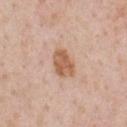Findings:
• notes · total-body-photography surveillance lesion; no biopsy
• anatomic site · the chest
• patient · male, approximately 50 years of age
• illumination · white-light
• size · ~3.5 mm (longest diameter)
• automated lesion analysis · a footprint of about 7.5 mm² and an outline eccentricity of about 0.75 (0 = round, 1 = elongated); a nevus-likeness score of about 80/100
• image · total-body-photography crop, ~15 mm field of view